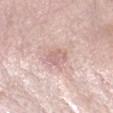The lesion was tiled from a total-body skin photograph and was not biopsied. A lesion tile, about 15 mm wide, cut from a 3D total-body photograph. A female subject, aged around 70. The lesion's longest dimension is about 2.5 mm. On the left forearm. The tile uses white-light illumination.